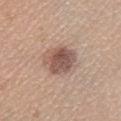<lesion>
<biopsy_status>not biopsied; imaged during a skin examination</biopsy_status>
<site>left lower leg</site>
<patient>
  <sex>female</sex>
  <age_approx>40</age_approx>
</patient>
<image>
  <source>total-body photography crop</source>
  <field_of_view_mm>15</field_of_view_mm>
</image>
<lighting>white-light</lighting>
<lesion_size>
  <long_diameter_mm_approx>4.5</long_diameter_mm_approx>
</lesion_size>
</lesion>